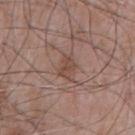Cropped from a total-body skin-imaging series; the visible field is about 15 mm.
The lesion is on the chest.
Automated image analysis of the tile measured a border-irregularity index near 4/10 and radial color variation of about 1.
A male patient, in their mid-60s.
Captured under white-light illumination.
The recorded lesion diameter is about 3 mm.